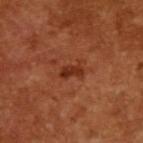follow-up = catalogued during a skin exam; not biopsied
lesion diameter = ≈2.5 mm
subject = male, in their mid- to late 60s
acquisition = ~15 mm crop, total-body skin-cancer survey